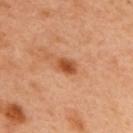Findings:
– workup · imaged on a skin check; not biopsied
– body site · the upper back
– image source · ~15 mm crop, total-body skin-cancer survey
– patient · male, roughly 50 years of age
– lesion diameter · ~2.5 mm (longest diameter)
– tile lighting · cross-polarized